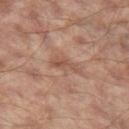biopsy_status: not biopsied; imaged during a skin examination
automated_metrics:
  area_mm2_approx: 4.5
  shape_asymmetry: 0.45
  border_irregularity_0_10: 5.5
  color_variation_0_10: 2.5
  peripheral_color_asymmetry: 1.0
image:
  source: total-body photography crop
  field_of_view_mm: 15
lesion_size:
  long_diameter_mm_approx: 4.0
site: left thigh
patient:
  sex: male
  age_approx: 65